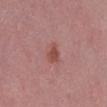The lesion was photographed on a routine skin check and not biopsied; there is no pathology result. The lesion is located on the leg. The subject is a female in their mid- to late 40s. A 15 mm close-up tile from a total-body photography series done for melanoma screening. Automated tile analysis of the lesion measured a mean CIELAB color near L≈49 a*≈26 b*≈25, roughly 9 lightness units darker than nearby skin, and a normalized lesion–skin contrast near 7.5. It also reported a border-irregularity rating of about 4/10, internal color variation of about 2 on a 0–10 scale, and a peripheral color-asymmetry measure near 1.